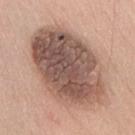| feature | finding |
|---|---|
| workup | no biopsy performed (imaged during a skin exam) |
| subject | male, about 40 years old |
| image | ~15 mm tile from a whole-body skin photo |
| automated metrics | a lesion color around L≈53 a*≈18 b*≈24 in CIELAB, about 16 CIELAB-L* units darker than the surrounding skin, and a lesion-to-skin contrast of about 10.5 (normalized; higher = more distinct) |
| site | the chest |
| lighting | white-light |